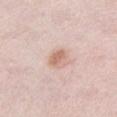The lesion is on the front of the torso. The subject is a female aged around 65. Approximately 2.5 mm at its widest. A roughly 15 mm field-of-view crop from a total-body skin photograph. Imaged with white-light lighting.